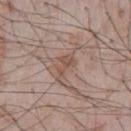Q: Was a biopsy performed?
A: imaged on a skin check; not biopsied
Q: What is the lesion's diameter?
A: about 3.5 mm
Q: Illumination type?
A: white-light illumination
Q: What is the imaging modality?
A: ~15 mm tile from a whole-body skin photo
Q: What did automated image analysis measure?
A: a lesion area of about 4 mm², an outline eccentricity of about 0.9 (0 = round, 1 = elongated), and two-axis asymmetry of about 0.5; an automated nevus-likeness rating near 0 out of 100
Q: What is the anatomic site?
A: the chest
Q: Patient demographics?
A: male, aged 63–67